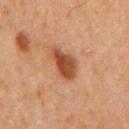Part of a total-body skin-imaging series; this lesion was reviewed on a skin check and was not flagged for biopsy.
Measured at roughly 4 mm in maximum diameter.
The lesion-visualizer software estimated a lesion area of about 7.5 mm² and two-axis asymmetry of about 0.3. It also reported a border-irregularity index near 3/10 and a color-variation rating of about 3.5/10. The software also gave a classifier nevus-likeness of about 95/100 and a lesion-detection confidence of about 100/100.
This is a cross-polarized tile.
The lesion is located on the mid back.
A male patient aged 63–67.
A 15 mm close-up extracted from a 3D total-body photography capture.